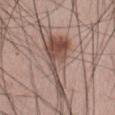Case summary:
• notes — imaged on a skin check; not biopsied